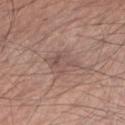| feature | finding |
|---|---|
| biopsy status | total-body-photography surveillance lesion; no biopsy |
| diameter | ≈4.5 mm |
| acquisition | ~15 mm tile from a whole-body skin photo |
| body site | the right forearm |
| subject | male, aged 68–72 |